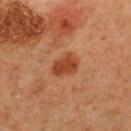{
  "biopsy_status": "not biopsied; imaged during a skin examination",
  "automated_metrics": {
    "lesion_detection_confidence_0_100": 100
  },
  "lesion_size": {
    "long_diameter_mm_approx": 3.0
  },
  "image": {
    "source": "total-body photography crop",
    "field_of_view_mm": 15
  },
  "site": "mid back",
  "patient": {
    "sex": "male",
    "age_approx": 65
  }
}